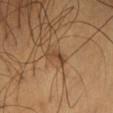Q: Is there a histopathology result?
A: no biopsy performed (imaged during a skin exam)
Q: Who is the patient?
A: male, aged 48 to 52
Q: How was the tile lit?
A: cross-polarized
Q: What is the imaging modality?
A: total-body-photography crop, ~15 mm field of view
Q: What is the anatomic site?
A: the chest
Q: What did automated image analysis measure?
A: an area of roughly 2.5 mm² and a symmetry-axis asymmetry near 0.7; a lesion–skin lightness drop of about 9 and a normalized border contrast of about 7.5; a border-irregularity rating of about 7.5/10, a within-lesion color-variation index near 0/10, and peripheral color asymmetry of about 0